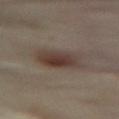| feature | finding |
|---|---|
| follow-up | no biopsy performed (imaged during a skin exam) |
| subject | female, aged 53–57 |
| lesion size | about 5 mm |
| lighting | cross-polarized illumination |
| acquisition | ~15 mm tile from a whole-body skin photo |
| body site | the front of the torso |
| automated lesion analysis | a footprint of about 12 mm², an outline eccentricity of about 0.8 (0 = round, 1 = elongated), and two-axis asymmetry of about 0.15; a within-lesion color-variation index near 5.5/10 and a peripheral color-asymmetry measure near 2; an automated nevus-likeness rating near 95 out of 100 and lesion-presence confidence of about 100/100 |